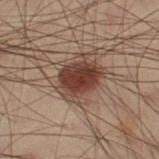| field | value |
|---|---|
| biopsy status | total-body-photography surveillance lesion; no biopsy |
| illumination | cross-polarized |
| size | ~5 mm (longest diameter) |
| body site | the right thigh |
| patient | male, in their mid- to late 50s |
| image source | ~15 mm crop, total-body skin-cancer survey |